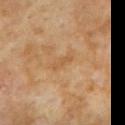Captured during whole-body skin photography for melanoma surveillance; the lesion was not biopsied.
A 15 mm close-up tile from a total-body photography series done for melanoma screening.
The recorded lesion diameter is about 3 mm.
Captured under cross-polarized illumination.
A male subject, aged 58–62.
From the abdomen.